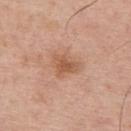No biopsy was performed on this lesion — it was imaged during a full skin examination and was not determined to be concerning. Longest diameter approximately 3.5 mm. A male subject, in their mid- to late 60s. On the upper back. Automated image analysis of the tile measured an average lesion color of about L≈56 a*≈22 b*≈33 (CIELAB) and about 9 CIELAB-L* units darker than the surrounding skin. The software also gave a classifier nevus-likeness of about 15/100 and a detector confidence of about 100 out of 100 that the crop contains a lesion. A roughly 15 mm field-of-view crop from a total-body skin photograph. Captured under white-light illumination.